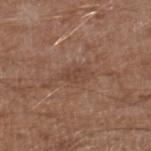Imaged during a routine full-body skin examination; the lesion was not biopsied and no histopathology is available.
A 15 mm close-up tile from a total-body photography series done for melanoma screening.
Located on the right lower leg.
An algorithmic analysis of the crop reported an area of roughly 3 mm², an outline eccentricity of about 0.85 (0 = round, 1 = elongated), and a shape-asymmetry score of about 0.3 (0 = symmetric). The analysis additionally found a lesion color around L≈43 a*≈19 b*≈27 in CIELAB, about 7 CIELAB-L* units darker than the surrounding skin, and a lesion-to-skin contrast of about 5.5 (normalized; higher = more distinct). The software also gave peripheral color asymmetry of about 0.
The tile uses white-light illumination.
A male patient, aged 63 to 67.